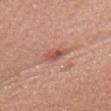workup=imaged on a skin check; not biopsied | subject=female, approximately 35 years of age | image source=15 mm crop, total-body photography | size=≈3 mm | site=the head or neck.